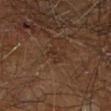biopsy status: imaged on a skin check; not biopsied | subject: male, aged 58 to 62 | automated metrics: an area of roughly 2.5 mm², an outline eccentricity of about 0.9 (0 = round, 1 = elongated), and a symmetry-axis asymmetry near 0.45; a lesion–skin lightness drop of about 5 and a normalized lesion–skin contrast near 5; border irregularity of about 4 on a 0–10 scale, a within-lesion color-variation index near 0/10, and radial color variation of about 0; an automated nevus-likeness rating near 0 out of 100 | body site: the right leg | lighting: cross-polarized | image source: total-body-photography crop, ~15 mm field of view | diameter: ~2.5 mm (longest diameter).